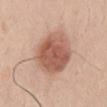Captured during whole-body skin photography for melanoma surveillance; the lesion was not biopsied.
A region of skin cropped from a whole-body photographic capture, roughly 15 mm wide.
Longest diameter approximately 6 mm.
Automated tile analysis of the lesion measured a classifier nevus-likeness of about 95/100 and a detector confidence of about 100 out of 100 that the crop contains a lesion.
On the mid back.
The subject is a male aged approximately 25.
The tile uses white-light illumination.